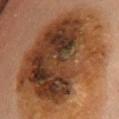This lesion was catalogued during total-body skin photography and was not selected for biopsy.
Located on the chest.
Cropped from a total-body skin-imaging series; the visible field is about 15 mm.
The tile uses cross-polarized illumination.
A female subject, aged approximately 60.
The recorded lesion diameter is about 14 mm.